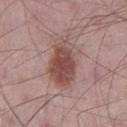No biopsy was performed on this lesion — it was imaged during a full skin examination and was not determined to be concerning.
Located on the left thigh.
A roughly 15 mm field-of-view crop from a total-body skin photograph.
This is a white-light tile.
A male patient approximately 65 years of age.